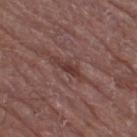follow-up: imaged on a skin check; not biopsied
image: total-body-photography crop, ~15 mm field of view
subject: male, roughly 75 years of age
image-analysis metrics: an area of roughly 3.5 mm² and a symmetry-axis asymmetry near 0.35; a border-irregularity rating of about 5/10, a color-variation rating of about 0.5/10, and peripheral color asymmetry of about 0
site: the right thigh